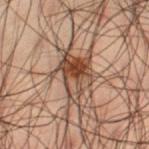Case summary:
– follow-up · total-body-photography surveillance lesion; no biopsy
– body site · the left thigh
– lighting · cross-polarized
– subject · male, in their 50s
– acquisition · ~15 mm tile from a whole-body skin photo
– diameter · about 5 mm